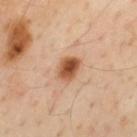On the mid back. Cropped from a whole-body photographic skin survey; the tile spans about 15 mm. A male patient approximately 55 years of age.